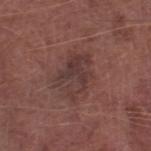Part of a total-body skin-imaging series; this lesion was reviewed on a skin check and was not flagged for biopsy.